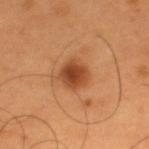Imaged during a routine full-body skin examination; the lesion was not biopsied and no histopathology is available.
The lesion's longest dimension is about 3 mm.
The lesion is located on the upper back.
The total-body-photography lesion software estimated a border-irregularity rating of about 1.5/10, internal color variation of about 4 on a 0–10 scale, and radial color variation of about 1.
A 15 mm crop from a total-body photograph taken for skin-cancer surveillance.
A male subject, aged around 55.
Captured under cross-polarized illumination.